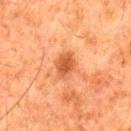Assessment:
The lesion was photographed on a routine skin check and not biopsied; there is no pathology result.
Image and clinical context:
Measured at roughly 3 mm in maximum diameter. Imaged with cross-polarized lighting. The lesion is located on the leg. The total-body-photography lesion software estimated an area of roughly 6 mm². And it measured a lesion color around L≈46 a*≈26 b*≈36 in CIELAB, a lesion–skin lightness drop of about 10, and a normalized lesion–skin contrast near 7.5. And it measured border irregularity of about 2 on a 0–10 scale, internal color variation of about 2 on a 0–10 scale, and radial color variation of about 0.5. A male subject, aged around 80. A roughly 15 mm field-of-view crop from a total-body skin photograph.